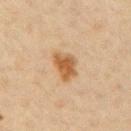The lesion was photographed on a routine skin check and not biopsied; there is no pathology result. The lesion is on the right upper arm. The patient is a female roughly 45 years of age. Cropped from a whole-body photographic skin survey; the tile spans about 15 mm.